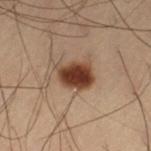Recorded during total-body skin imaging; not selected for excision or biopsy.
A region of skin cropped from a whole-body photographic capture, roughly 15 mm wide.
From the left thigh.
The patient is a male aged 53–57.